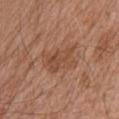imaging modality: ~15 mm tile from a whole-body skin photo | patient: male, aged 73–77 | location: the abdomen | diameter: ~4.5 mm (longest diameter) | illumination: white-light | automated metrics: a lesion color around L≈48 a*≈22 b*≈31 in CIELAB, roughly 7 lightness units darker than nearby skin, and a normalized lesion–skin contrast near 5.5.